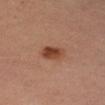The lesion was tiled from a total-body skin photograph and was not biopsied. A female subject, in their mid-30s. The lesion is located on the arm. Captured under cross-polarized illumination. A region of skin cropped from a whole-body photographic capture, roughly 15 mm wide.